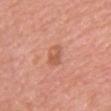workup — catalogued during a skin exam; not biopsied | size — ≈2.5 mm | automated metrics — a footprint of about 4.5 mm² and an outline eccentricity of about 0.75 (0 = round, 1 = elongated) | anatomic site — the chest | image source — 15 mm crop, total-body photography | patient — male, about 55 years old | lighting — white-light illumination.